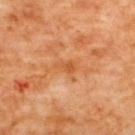A female subject in their mid- to late 60s. A 15 mm close-up tile from a total-body photography series done for melanoma screening. On the back. The tile uses cross-polarized illumination.